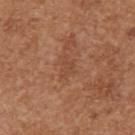biopsy status: total-body-photography surveillance lesion; no biopsy | location: the right upper arm | lesion diameter: about 3 mm | acquisition: total-body-photography crop, ~15 mm field of view | patient: female, in their 40s | TBP lesion metrics: a footprint of about 3 mm², an outline eccentricity of about 0.8 (0 = round, 1 = elongated), and a shape-asymmetry score of about 0.5 (0 = symmetric).